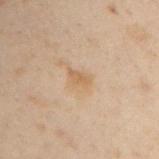workup: catalogued during a skin exam; not biopsied
location: the left upper arm
patient: male, approximately 50 years of age
acquisition: total-body-photography crop, ~15 mm field of view